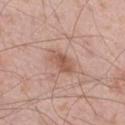Clinical impression: No biopsy was performed on this lesion — it was imaged during a full skin examination and was not determined to be concerning. Clinical summary: A 15 mm close-up tile from a total-body photography series done for melanoma screening. From the right thigh. Measured at roughly 3.5 mm in maximum diameter. A male subject, aged around 55. An algorithmic analysis of the crop reported a lesion area of about 6.5 mm², a shape eccentricity near 0.8, and a symmetry-axis asymmetry near 0.2. It also reported a lesion color around L≈56 a*≈21 b*≈28 in CIELAB, a lesion–skin lightness drop of about 9, and a normalized lesion–skin contrast near 6.5. It also reported a border-irregularity rating of about 2/10, a within-lesion color-variation index near 3.5/10, and peripheral color asymmetry of about 1.